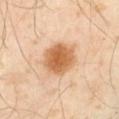| key | value |
|---|---|
| biopsy status | no biopsy performed (imaged during a skin exam) |
| size | ~4.5 mm (longest diameter) |
| image-analysis metrics | roughly 14 lightness units darker than nearby skin and a lesion-to-skin contrast of about 10 (normalized; higher = more distinct); a border-irregularity index near 1.5/10, a color-variation rating of about 3.5/10, and radial color variation of about 1; a classifier nevus-likeness of about 100/100 and a lesion-detection confidence of about 100/100 |
| site | the left thigh |
| patient | male, aged 43–47 |
| illumination | cross-polarized |
| image source | ~15 mm tile from a whole-body skin photo |